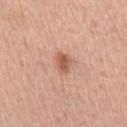Part of a total-body skin-imaging series; this lesion was reviewed on a skin check and was not flagged for biopsy. The total-body-photography lesion software estimated an area of roughly 4 mm² and a shape-asymmetry score of about 0.3 (0 = symmetric). A male subject, aged around 50. A 15 mm crop from a total-body photograph taken for skin-cancer surveillance. Longest diameter approximately 2.5 mm. The lesion is located on the right upper arm.